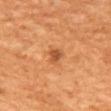No biopsy was performed on this lesion — it was imaged during a full skin examination and was not determined to be concerning. A roughly 15 mm field-of-view crop from a total-body skin photograph. A female subject aged around 65. Automated image analysis of the tile measured a footprint of about 2.5 mm², an outline eccentricity of about 0.65 (0 = round, 1 = elongated), and two-axis asymmetry of about 0.3. The analysis additionally found a lesion–skin lightness drop of about 11. The software also gave a border-irregularity rating of about 2.5/10, internal color variation of about 1 on a 0–10 scale, and a peripheral color-asymmetry measure near 0.5. On the upper back.